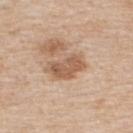Assessment:
This lesion was catalogued during total-body skin photography and was not selected for biopsy.
Acquisition and patient details:
This is a white-light tile. A 15 mm close-up extracted from a 3D total-body photography capture. The subject is a male about 60 years old. From the upper back. The lesion-visualizer software estimated about 12 CIELAB-L* units darker than the surrounding skin. And it measured border irregularity of about 3 on a 0–10 scale and internal color variation of about 3 on a 0–10 scale. The analysis additionally found an automated nevus-likeness rating near 55 out of 100 and a lesion-detection confidence of about 100/100.